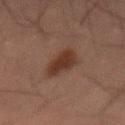Q: Was a biopsy performed?
A: no biopsy performed (imaged during a skin exam)
Q: Lesion size?
A: about 4.5 mm
Q: What is the imaging modality?
A: 15 mm crop, total-body photography
Q: Who is the patient?
A: male, approximately 55 years of age
Q: Lesion location?
A: the abdomen
Q: Illumination type?
A: cross-polarized illumination